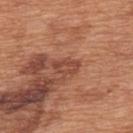Part of a total-body skin-imaging series; this lesion was reviewed on a skin check and was not flagged for biopsy.
On the back.
Captured under white-light illumination.
A close-up tile cropped from a whole-body skin photograph, about 15 mm across.
A male patient aged 63–67.
About 3.5 mm across.